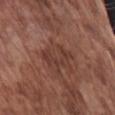Q: Is there a histopathology result?
A: no biopsy performed (imaged during a skin exam)
Q: How large is the lesion?
A: about 4.5 mm
Q: Who is the patient?
A: male, in their mid- to late 70s
Q: Illumination type?
A: white-light illumination
Q: What did automated image analysis measure?
A: a footprint of about 7.5 mm² and an outline eccentricity of about 0.85 (0 = round, 1 = elongated); a border-irregularity index near 6.5/10, internal color variation of about 3 on a 0–10 scale, and a peripheral color-asymmetry measure near 1; an automated nevus-likeness rating near 0 out of 100 and a detector confidence of about 100 out of 100 that the crop contains a lesion
Q: How was this image acquired?
A: ~15 mm tile from a whole-body skin photo
Q: Lesion location?
A: the arm